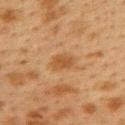Image and clinical context:
Measured at roughly 3 mm in maximum diameter. The subject is a female approximately 40 years of age. The lesion is on the upper back. Cropped from a whole-body photographic skin survey; the tile spans about 15 mm. This is a cross-polarized tile.